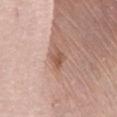workup = total-body-photography surveillance lesion; no biopsy
image = 15 mm crop, total-body photography
location = the chest
size = ~3 mm (longest diameter)
patient = female, in their mid-60s
illumination = white-light